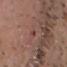This lesion was catalogued during total-body skin photography and was not selected for biopsy. A 15 mm crop from a total-body photograph taken for skin-cancer surveillance. The total-body-photography lesion software estimated an area of roughly 1.5 mm², an outline eccentricity of about 0.95 (0 = round, 1 = elongated), and a symmetry-axis asymmetry near 0.4. And it measured an automated nevus-likeness rating near 0 out of 100 and lesion-presence confidence of about 95/100. A male patient, aged 53 to 57. Located on the chest. Approximately 2.5 mm at its widest.